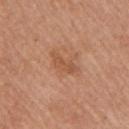<lesion>
<biopsy_status>not biopsied; imaged during a skin examination</biopsy_status>
<lesion_size>
  <long_diameter_mm_approx>3.5</long_diameter_mm_approx>
</lesion_size>
<lighting>white-light</lighting>
<image>
  <source>total-body photography crop</source>
  <field_of_view_mm>15</field_of_view_mm>
</image>
<patient>
  <sex>female</sex>
  <age_approx>60</age_approx>
</patient>
<site>arm</site>
<automated_metrics>
  <border_irregularity_0_10>4.5</border_irregularity_0_10>
  <color_variation_0_10>1.5</color_variation_0_10>
  <peripheral_color_asymmetry>0.5</peripheral_color_asymmetry>
  <lesion_detection_confidence_0_100>100</lesion_detection_confidence_0_100>
</automated_metrics>
</lesion>